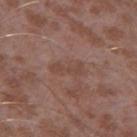The lesion was tiled from a total-body skin photograph and was not biopsied.
A male patient, aged approximately 45.
On the left thigh.
A 15 mm close-up tile from a total-body photography series done for melanoma screening.